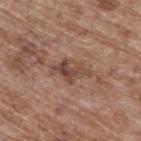Part of a total-body skin-imaging series; this lesion was reviewed on a skin check and was not flagged for biopsy.
The lesion's longest dimension is about 4.5 mm.
Imaged with white-light lighting.
An algorithmic analysis of the crop reported a lesion area of about 8 mm² and two-axis asymmetry of about 0.25. It also reported a border-irregularity rating of about 3.5/10, internal color variation of about 6 on a 0–10 scale, and a peripheral color-asymmetry measure near 2.
A male subject in their 70s.
A lesion tile, about 15 mm wide, cut from a 3D total-body photograph.
On the upper back.